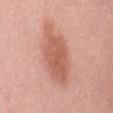Recorded during total-body skin imaging; not selected for excision or biopsy.
Imaged with white-light lighting.
A female patient approximately 65 years of age.
An algorithmic analysis of the crop reported a normalized border contrast of about 7.5. It also reported a border-irregularity index near 3/10, internal color variation of about 3 on a 0–10 scale, and radial color variation of about 1. The analysis additionally found a nevus-likeness score of about 95/100 and lesion-presence confidence of about 100/100.
About 8.5 mm across.
A region of skin cropped from a whole-body photographic capture, roughly 15 mm wide.
The lesion is located on the mid back.